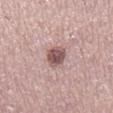biopsy status: imaged on a skin check; not biopsied | acquisition: ~15 mm crop, total-body skin-cancer survey | subject: female, aged 38 to 42 | body site: the right lower leg.